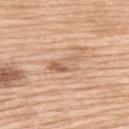This lesion was catalogued during total-body skin photography and was not selected for biopsy.
The subject is a female approximately 55 years of age.
The lesion is located on the upper back.
A 15 mm crop from a total-body photograph taken for skin-cancer surveillance.